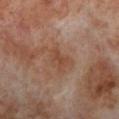Q: Is there a histopathology result?
A: catalogued during a skin exam; not biopsied
Q: What lighting was used for the tile?
A: cross-polarized illumination
Q: What is the imaging modality?
A: 15 mm crop, total-body photography
Q: Lesion size?
A: ~3.5 mm (longest diameter)
Q: Lesion location?
A: the left lower leg
Q: Who is the patient?
A: male, in their 70s